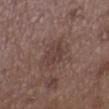follow-up: catalogued during a skin exam; not biopsied | location: the upper back | patient: female, in their mid- to late 30s | image source: ~15 mm tile from a whole-body skin photo | tile lighting: white-light | size: about 4 mm.